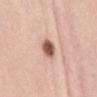Impression: The lesion was tiled from a total-body skin photograph and was not biopsied. Clinical summary: Located on the abdomen. A lesion tile, about 15 mm wide, cut from a 3D total-body photograph. A female subject in their mid- to late 30s. The lesion-visualizer software estimated an eccentricity of roughly 0.55 and two-axis asymmetry of about 0.2. It also reported roughly 19 lightness units darker than nearby skin. The tile uses white-light illumination.